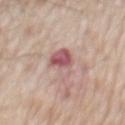Notes:
* biopsy status · imaged on a skin check; not biopsied
* lesion size · about 5.5 mm
* image source · 15 mm crop, total-body photography
* image-analysis metrics · a footprint of about 8 mm², an eccentricity of roughly 0.9, and two-axis asymmetry of about 0.35; an average lesion color of about L≈57 a*≈23 b*≈20 (CIELAB), a lesion–skin lightness drop of about 12, and a normalized border contrast of about 8.5; border irregularity of about 4.5 on a 0–10 scale, internal color variation of about 5.5 on a 0–10 scale, and peripheral color asymmetry of about 1.5
* patient · male, approximately 65 years of age
* location · the mid back